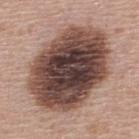No biopsy was performed on this lesion — it was imaged during a full skin examination and was not determined to be concerning.
Automated image analysis of the tile measured a lesion area of about 65 mm², an eccentricity of roughly 0.7, and a shape-asymmetry score of about 0.1 (0 = symmetric).
The patient is a male about 55 years old.
The tile uses white-light illumination.
Longest diameter approximately 11 mm.
A 15 mm close-up tile from a total-body photography series done for melanoma screening.